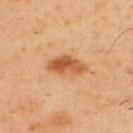{
  "biopsy_status": "not biopsied; imaged during a skin examination",
  "lesion_size": {
    "long_diameter_mm_approx": 4.5
  },
  "site": "back",
  "patient": {
    "sex": "male",
    "age_approx": 55
  },
  "image": {
    "source": "total-body photography crop",
    "field_of_view_mm": 15
  },
  "automated_metrics": {
    "cielab_L": 55,
    "cielab_a": 24,
    "cielab_b": 40,
    "vs_skin_darker_L": 12.0,
    "vs_skin_contrast_norm": 8.5,
    "nevus_likeness_0_100": 95,
    "lesion_detection_confidence_0_100": 100
  }
}